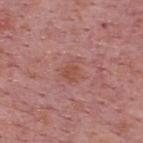Q: Was a biopsy performed?
A: catalogued during a skin exam; not biopsied
Q: How was this image acquired?
A: 15 mm crop, total-body photography
Q: Where on the body is the lesion?
A: the upper back
Q: What are the patient's age and sex?
A: male, about 75 years old